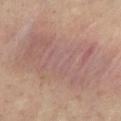Case summary:
• patient · male, in their mid-30s
• image source · 15 mm crop, total-body photography
• lighting · cross-polarized
• anatomic site · the back
• lesion size · about 11.5 mm